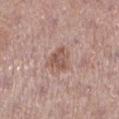workup — total-body-photography surveillance lesion; no biopsy | acquisition — 15 mm crop, total-body photography | subject — female, aged approximately 40 | location — the left lower leg.